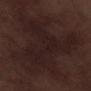Captured during whole-body skin photography for melanoma surveillance; the lesion was not biopsied.
A 15 mm close-up tile from a total-body photography series done for melanoma screening.
The subject is a male roughly 70 years of age.
Located on the right lower leg.
The recorded lesion diameter is about 10 mm.
Automated tile analysis of the lesion measured a lesion area of about 48 mm², a shape eccentricity near 0.5, and a symmetry-axis asymmetry near 0.6. It also reported a lesion color around L≈19 a*≈14 b*≈15 in CIELAB, roughly 4 lightness units darker than nearby skin, and a normalized lesion–skin contrast near 6.5.